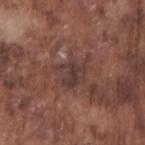Recorded during total-body skin imaging; not selected for excision or biopsy. Located on the right upper arm. A lesion tile, about 15 mm wide, cut from a 3D total-body photograph. The lesion's longest dimension is about 4.5 mm. The patient is a male in their mid- to late 70s.